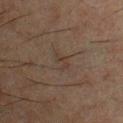Case summary:
– acquisition — ~15 mm tile from a whole-body skin photo
– body site — the chest
– patient — male, approximately 50 years of age